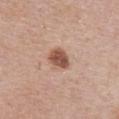Recorded during total-body skin imaging; not selected for excision or biopsy.
A female subject in their mid- to late 60s.
An algorithmic analysis of the crop reported an area of roughly 6 mm², a shape eccentricity near 0.6, and two-axis asymmetry of about 0.2.
A lesion tile, about 15 mm wide, cut from a 3D total-body photograph.
The lesion is located on the chest.